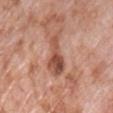Assessment:
No biopsy was performed on this lesion — it was imaged during a full skin examination and was not determined to be concerning.